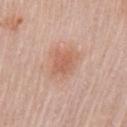Q: Was this lesion biopsied?
A: no biopsy performed (imaged during a skin exam)
Q: What kind of image is this?
A: 15 mm crop, total-body photography
Q: Lesion size?
A: ≈3 mm
Q: Where on the body is the lesion?
A: the arm
Q: What did automated image analysis measure?
A: a footprint of about 7.5 mm², an outline eccentricity of about 0.3 (0 = round, 1 = elongated), and a symmetry-axis asymmetry near 0.25; a mean CIELAB color near L≈60 a*≈23 b*≈30, roughly 8 lightness units darker than nearby skin, and a lesion-to-skin contrast of about 6 (normalized; higher = more distinct); a border-irregularity index near 2.5/10, internal color variation of about 2 on a 0–10 scale, and peripheral color asymmetry of about 0.5; a detector confidence of about 100 out of 100 that the crop contains a lesion
Q: Illumination type?
A: white-light
Q: Patient demographics?
A: female, about 65 years old